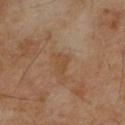  biopsy_status: not biopsied; imaged during a skin examination
  image:
    source: total-body photography crop
    field_of_view_mm: 15
  automated_metrics:
    eccentricity: 0.8
    shape_asymmetry: 0.3
  patient:
    sex: male
    age_approx: 55
  site: back
  lesion_size:
    long_diameter_mm_approx: 2.5
  lighting: cross-polarized